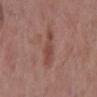| key | value |
|---|---|
| follow-up | catalogued during a skin exam; not biopsied |
| image | 15 mm crop, total-body photography |
| diameter | about 4 mm |
| anatomic site | the mid back |
| tile lighting | white-light illumination |
| subject | male, aged 58–62 |
| TBP lesion metrics | a lesion–skin lightness drop of about 8; a border-irregularity index near 5/10 and radial color variation of about 0.5 |